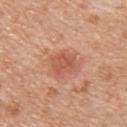{
  "biopsy_status": "not biopsied; imaged during a skin examination",
  "lesion_size": {
    "long_diameter_mm_approx": 3.5
  },
  "image": {
    "source": "total-body photography crop",
    "field_of_view_mm": 15
  },
  "patient": {
    "sex": "female",
    "age_approx": 55
  },
  "site": "upper back",
  "lighting": "white-light",
  "automated_metrics": {
    "area_mm2_approx": 8.0,
    "eccentricity": 0.6,
    "shape_asymmetry": 0.2,
    "cielab_L": 56,
    "cielab_a": 27,
    "cielab_b": 33,
    "vs_skin_darker_L": 9.0,
    "vs_skin_contrast_norm": 6.0,
    "color_variation_0_10": 2.0,
    "peripheral_color_asymmetry": 0.5,
    "nevus_likeness_0_100": 85
  }
}